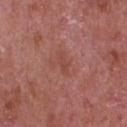Clinical impression: This lesion was catalogued during total-body skin photography and was not selected for biopsy. Context: A region of skin cropped from a whole-body photographic capture, roughly 15 mm wide. The total-body-photography lesion software estimated a footprint of about 2.5 mm² and a shape-asymmetry score of about 0.3 (0 = symmetric). And it measured a nevus-likeness score of about 0/100 and a detector confidence of about 100 out of 100 that the crop contains a lesion. Located on the chest. A male subject aged approximately 65.